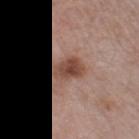A 15 mm close-up tile from a total-body photography series done for melanoma screening. The tile uses white-light illumination. A female subject roughly 40 years of age. The recorded lesion diameter is about 4 mm. An algorithmic analysis of the crop reported an area of roughly 7.5 mm², an outline eccentricity of about 0.75 (0 = round, 1 = elongated), and a shape-asymmetry score of about 0.2 (0 = symmetric). It also reported a mean CIELAB color near L≈47 a*≈20 b*≈26, roughly 12 lightness units darker than nearby skin, and a lesion-to-skin contrast of about 9 (normalized; higher = more distinct). And it measured a border-irregularity index near 2/10, internal color variation of about 4 on a 0–10 scale, and radial color variation of about 1. And it measured an automated nevus-likeness rating near 90 out of 100 and a detector confidence of about 100 out of 100 that the crop contains a lesion. On the left thigh.